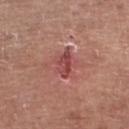Notes:
– workup: no biopsy performed (imaged during a skin exam)
– site: the left lower leg
– size: ≈4 mm
– imaging modality: ~15 mm crop, total-body skin-cancer survey
– patient: female, roughly 75 years of age
– lighting: white-light illumination
– image-analysis metrics: an average lesion color of about L≈49 a*≈28 b*≈24 (CIELAB), a lesion–skin lightness drop of about 8, and a normalized lesion–skin contrast near 6; a border-irregularity rating of about 4.5/10 and radial color variation of about 2.5; a nevus-likeness score of about 0/100 and lesion-presence confidence of about 100/100